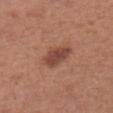Acquisition and patient details: Approximately 4 mm at its widest. Cropped from a total-body skin-imaging series; the visible field is about 15 mm. The lesion is located on the chest. A female subject, approximately 50 years of age.